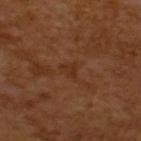Impression:
Recorded during total-body skin imaging; not selected for excision or biopsy.
Context:
About 3 mm across. This image is a 15 mm lesion crop taken from a total-body photograph. The total-body-photography lesion software estimated an area of roughly 3.5 mm², a shape eccentricity near 0.7, and a shape-asymmetry score of about 0.5 (0 = symmetric). The software also gave a lesion color around L≈30 a*≈20 b*≈30 in CIELAB, roughly 5 lightness units darker than nearby skin, and a normalized border contrast of about 5. Captured under cross-polarized illumination. The patient is a male aged approximately 65.